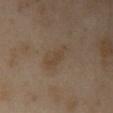| field | value |
|---|---|
| notes | no biopsy performed (imaged during a skin exam) |
| subject | female, aged around 40 |
| imaging modality | total-body-photography crop, ~15 mm field of view |
| body site | the left arm |